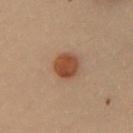notes: total-body-photography surveillance lesion; no biopsy
lesion diameter: about 3.5 mm
anatomic site: the chest
illumination: cross-polarized illumination
image: ~15 mm tile from a whole-body skin photo
subject: female, aged 28 to 32
automated metrics: a footprint of about 7 mm² and a shape eccentricity near 0.6; a lesion color around L≈36 a*≈19 b*≈27 in CIELAB, about 10 CIELAB-L* units darker than the surrounding skin, and a normalized lesion–skin contrast near 10; a within-lesion color-variation index near 2.5/10 and a peripheral color-asymmetry measure near 1; lesion-presence confidence of about 100/100